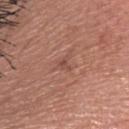Part of a total-body skin-imaging series; this lesion was reviewed on a skin check and was not flagged for biopsy. The lesion is on the head or neck. Cropped from a total-body skin-imaging series; the visible field is about 15 mm. Imaged with white-light lighting. The subject is a female about 50 years old. Measured at roughly 2 mm in maximum diameter.